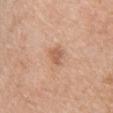  biopsy_status: not biopsied; imaged during a skin examination
  image:
    source: total-body photography crop
    field_of_view_mm: 15
  site: chest
  lighting: white-light
  patient:
    sex: male
    age_approx: 65